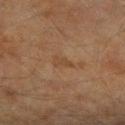<record>
<biopsy_status>not biopsied; imaged during a skin examination</biopsy_status>
<automated_metrics>
  <vs_skin_contrast_norm>5.5</vs_skin_contrast_norm>
  <border_irregularity_0_10>4.0</border_irregularity_0_10>
  <color_variation_0_10>0.0</color_variation_0_10>
  <peripheral_color_asymmetry>0.0</peripheral_color_asymmetry>
</automated_metrics>
<lesion_size>
  <long_diameter_mm_approx>2.5</long_diameter_mm_approx>
</lesion_size>
<lighting>cross-polarized</lighting>
<patient>
  <sex>female</sex>
  <age_approx>60</age_approx>
</patient>
<image>
  <source>total-body photography crop</source>
  <field_of_view_mm>15</field_of_view_mm>
</image>
<site>left forearm</site>
</record>